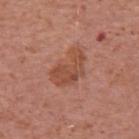Q: Was a biopsy performed?
A: total-body-photography surveillance lesion; no biopsy
Q: What kind of image is this?
A: ~15 mm tile from a whole-body skin photo
Q: Lesion size?
A: ~4.5 mm (longest diameter)
Q: What is the anatomic site?
A: the upper back
Q: Patient demographics?
A: male, about 70 years old
Q: Illumination type?
A: white-light illumination